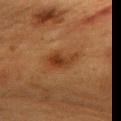workup = total-body-photography surveillance lesion; no biopsy
subject = female, aged 53–57
anatomic site = the chest
size = ~3.5 mm (longest diameter)
image source = total-body-photography crop, ~15 mm field of view